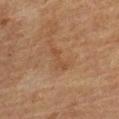Case summary:
* workup: imaged on a skin check; not biopsied
* automated metrics: a footprint of about 3 mm² and a shape-asymmetry score of about 0.55 (0 = symmetric); a mean CIELAB color near L≈41 a*≈18 b*≈30, about 5 CIELAB-L* units darker than the surrounding skin, and a normalized lesion–skin contrast near 5; border irregularity of about 7.5 on a 0–10 scale and peripheral color asymmetry of about 0
* subject: male, approximately 85 years of age or older
* lesion diameter: about 3.5 mm
* image: 15 mm crop, total-body photography
* anatomic site: the right lower leg